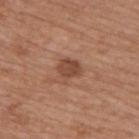| field | value |
|---|---|
| workup | catalogued during a skin exam; not biopsied |
| lesion diameter | about 3 mm |
| subject | male, about 65 years old |
| body site | the upper back |
| image source | 15 mm crop, total-body photography |
| image-analysis metrics | a border-irregularity rating of about 3.5/10 and a peripheral color-asymmetry measure near 1; a classifier nevus-likeness of about 30/100 and lesion-presence confidence of about 100/100 |